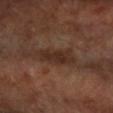Q: Is there a histopathology result?
A: total-body-photography surveillance lesion; no biopsy
Q: Patient demographics?
A: female, aged around 60
Q: What lighting was used for the tile?
A: cross-polarized illumination
Q: Automated lesion metrics?
A: a lesion–skin lightness drop of about 8 and a normalized border contrast of about 7.5; border irregularity of about 3 on a 0–10 scale, a within-lesion color-variation index near 2/10, and a peripheral color-asymmetry measure near 0.5; lesion-presence confidence of about 80/100
Q: What kind of image is this?
A: total-body-photography crop, ~15 mm field of view
Q: Lesion size?
A: ~5 mm (longest diameter)
Q: Lesion location?
A: the left arm